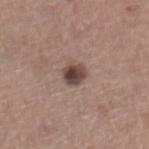workup — no biopsy performed (imaged during a skin exam)
image-analysis metrics — a lesion area of about 5 mm²; an average lesion color of about L≈44 a*≈17 b*≈21 (CIELAB), about 14 CIELAB-L* units darker than the surrounding skin, and a normalized border contrast of about 11; a classifier nevus-likeness of about 90/100 and lesion-presence confidence of about 100/100
lesion diameter — ≈2.5 mm
subject — male, aged 63–67
imaging modality — ~15 mm crop, total-body skin-cancer survey
location — the left lower leg
lighting — white-light illumination